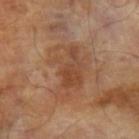Impression: Recorded during total-body skin imaging; not selected for excision or biopsy. Clinical summary: Located on the left forearm. Cropped from a whole-body photographic skin survey; the tile spans about 15 mm. A male patient aged 68 to 72. The recorded lesion diameter is about 6.5 mm.